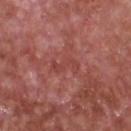Imaged during a routine full-body skin examination; the lesion was not biopsied and no histopathology is available. The lesion is on the chest. A male subject aged 63–67. Measured at roughly 3.5 mm in maximum diameter. Imaged with white-light lighting. A lesion tile, about 15 mm wide, cut from a 3D total-body photograph.